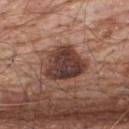A male subject aged 78–82.
On the back.
A close-up tile cropped from a whole-body skin photograph, about 15 mm across.
About 5 mm across.